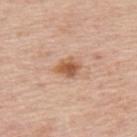Captured during whole-body skin photography for melanoma surveillance; the lesion was not biopsied.
The patient is a male about 75 years old.
Measured at roughly 3 mm in maximum diameter.
A 15 mm close-up extracted from a 3D total-body photography capture.
This is a white-light tile.
The lesion is on the upper back.